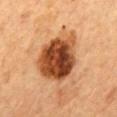| feature | finding |
|---|---|
| subject | female, aged 58–62 |
| tile lighting | cross-polarized |
| image source | 15 mm crop, total-body photography |
| location | the mid back |
| TBP lesion metrics | a footprint of about 25 mm², an outline eccentricity of about 0.65 (0 = round, 1 = elongated), and a shape-asymmetry score of about 0.2 (0 = symmetric); an average lesion color of about L≈42 a*≈24 b*≈35 (CIELAB) and about 19 CIELAB-L* units darker than the surrounding skin |